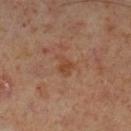This lesion was catalogued during total-body skin photography and was not selected for biopsy. The lesion is located on the left lower leg. The tile uses cross-polarized illumination. This image is a 15 mm lesion crop taken from a total-body photograph. A male patient aged approximately 60. Measured at roughly 2.5 mm in maximum diameter.